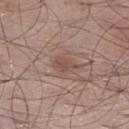Longest diameter approximately 3 mm.
This is a white-light tile.
A lesion tile, about 15 mm wide, cut from a 3D total-body photograph.
From the right lower leg.
The patient is a male about 55 years old.